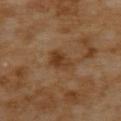lighting: cross-polarized illumination; image source: total-body-photography crop, ~15 mm field of view; lesion size: ~3.5 mm (longest diameter); anatomic site: the upper back; subject: female, aged approximately 55.